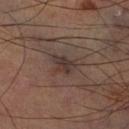The lesion was tiled from a total-body skin photograph and was not biopsied. Cropped from a whole-body photographic skin survey; the tile spans about 15 mm. The lesion is on the leg.